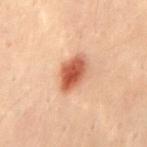biopsy status: total-body-photography surveillance lesion; no biopsy | subject: male, approximately 30 years of age | body site: the mid back | image source: 15 mm crop, total-body photography | lighting: cross-polarized illumination | TBP lesion metrics: an eccentricity of roughly 0.8 and a symmetry-axis asymmetry near 0.15; a mean CIELAB color near L≈47 a*≈24 b*≈30 and a normalized border contrast of about 10.5; a border-irregularity index near 1.5/10 and a peripheral color-asymmetry measure near 1.5 | diameter: about 4.5 mm.